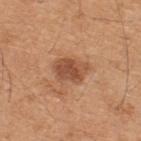Assessment: Imaged during a routine full-body skin examination; the lesion was not biopsied and no histopathology is available. Image and clinical context: A male patient, aged 43–47. From the back. A lesion tile, about 15 mm wide, cut from a 3D total-body photograph.